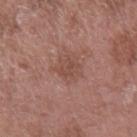Imaged during a routine full-body skin examination; the lesion was not biopsied and no histopathology is available. The lesion is located on the left forearm. Measured at roughly 3 mm in maximum diameter. A 15 mm crop from a total-body photograph taken for skin-cancer surveillance. A male subject, aged approximately 75. This is a white-light tile.